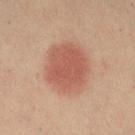Captured during whole-body skin photography for melanoma surveillance; the lesion was not biopsied. The lesion is on the left leg. The tile uses cross-polarized illumination. The recorded lesion diameter is about 6 mm. A region of skin cropped from a whole-body photographic capture, roughly 15 mm wide. The patient is a female in their mid- to late 30s. Automated tile analysis of the lesion measured a mean CIELAB color near L≈57 a*≈26 b*≈30, roughly 11 lightness units darker than nearby skin, and a lesion-to-skin contrast of about 7 (normalized; higher = more distinct). It also reported an automated nevus-likeness rating near 100 out of 100.